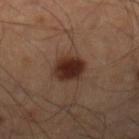follow-up — no biopsy performed (imaged during a skin exam) | image-analysis metrics — an area of roughly 8.5 mm², an outline eccentricity of about 0.6 (0 = round, 1 = elongated), and a shape-asymmetry score of about 0.15 (0 = symmetric); lesion-presence confidence of about 100/100 | body site — the left leg | acquisition — 15 mm crop, total-body photography | subject — male, in their 50s.